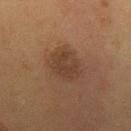follow-up: total-body-photography surveillance lesion; no biopsy
body site: the back
imaging modality: total-body-photography crop, ~15 mm field of view
patient: female, aged approximately 50